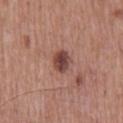Impression:
This lesion was catalogued during total-body skin photography and was not selected for biopsy.
Acquisition and patient details:
A lesion tile, about 15 mm wide, cut from a 3D total-body photograph. A male patient aged around 60. The lesion is on the mid back.